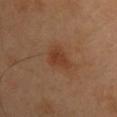Impression:
No biopsy was performed on this lesion — it was imaged during a full skin examination and was not determined to be concerning.
Context:
The patient is a male aged 38 to 42. Imaged with cross-polarized lighting. Approximately 3 mm at its widest. Located on the chest. This image is a 15 mm lesion crop taken from a total-body photograph. An algorithmic analysis of the crop reported an area of roughly 6 mm² and an outline eccentricity of about 0.65 (0 = round, 1 = elongated). And it measured an average lesion color of about L≈39 a*≈22 b*≈32 (CIELAB), about 7 CIELAB-L* units darker than the surrounding skin, and a normalized border contrast of about 6.5. The software also gave internal color variation of about 2.5 on a 0–10 scale and radial color variation of about 1. And it measured lesion-presence confidence of about 100/100.